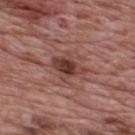Image and clinical context: A male subject aged approximately 70. This is a white-light tile. An algorithmic analysis of the crop reported an area of roughly 7.5 mm², an outline eccentricity of about 0.75 (0 = round, 1 = elongated), and two-axis asymmetry of about 0.3. It also reported border irregularity of about 3 on a 0–10 scale, a color-variation rating of about 4.5/10, and peripheral color asymmetry of about 1.5. The lesion is on the upper back. The lesion's longest dimension is about 4 mm. Cropped from a whole-body photographic skin survey; the tile spans about 15 mm.